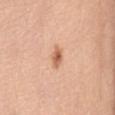{
  "biopsy_status": "not biopsied; imaged during a skin examination",
  "patient": {
    "sex": "female",
    "age_approx": 75
  },
  "image": {
    "source": "total-body photography crop",
    "field_of_view_mm": 15
  },
  "site": "front of the torso"
}